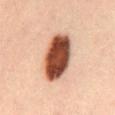The lesion was photographed on a routine skin check and not biopsied; there is no pathology result.
A 15 mm close-up tile from a total-body photography series done for melanoma screening.
A male patient approximately 30 years of age.
Longest diameter approximately 6.5 mm.
Located on the abdomen.
Captured under cross-polarized illumination.